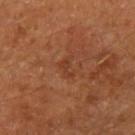The lesion is located on the left upper arm.
A male subject aged 63 to 67.
A region of skin cropped from a whole-body photographic capture, roughly 15 mm wide.